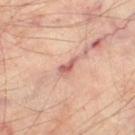Clinical impression: The lesion was photographed on a routine skin check and not biopsied; there is no pathology result. Context: On the left thigh. This is a cross-polarized tile. A region of skin cropped from a whole-body photographic capture, roughly 15 mm wide. The recorded lesion diameter is about 3 mm. Automated tile analysis of the lesion measured an outline eccentricity of about 0.9 (0 = round, 1 = elongated) and a symmetry-axis asymmetry near 0.45. It also reported a lesion color around L≈60 a*≈26 b*≈26 in CIELAB, roughly 12 lightness units darker than nearby skin, and a lesion-to-skin contrast of about 7.5 (normalized; higher = more distinct). And it measured a border-irregularity index near 5/10. A patient aged 53–57.